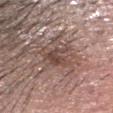{
  "patient": {
    "sex": "male",
    "age_approx": 55
  },
  "automated_metrics": {
    "cielab_L": 46,
    "cielab_a": 17,
    "cielab_b": 23,
    "vs_skin_darker_L": 9.0,
    "nevus_likeness_0_100": 20,
    "lesion_detection_confidence_0_100": 95
  },
  "image": {
    "source": "total-body photography crop",
    "field_of_view_mm": 15
  },
  "lesion_size": {
    "long_diameter_mm_approx": 4.0
  },
  "site": "head or neck"
}